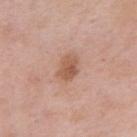This lesion was catalogued during total-body skin photography and was not selected for biopsy. The lesion is on the chest. Imaged with white-light lighting. A 15 mm close-up extracted from a 3D total-body photography capture. The subject is a male about 55 years old.